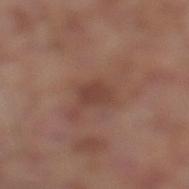{
  "biopsy_status": "not biopsied; imaged during a skin examination",
  "lesion_size": {
    "long_diameter_mm_approx": 3.0
  },
  "patient": {
    "sex": "male",
    "age_approx": 80
  },
  "site": "left lower leg",
  "automated_metrics": {
    "vs_skin_darker_L": 7.0,
    "vs_skin_contrast_norm": 6.0,
    "border_irregularity_0_10": 2.5,
    "color_variation_0_10": 1.5,
    "peripheral_color_asymmetry": 0.5
  },
  "lighting": "white-light",
  "image": {
    "source": "total-body photography crop",
    "field_of_view_mm": 15
  }
}